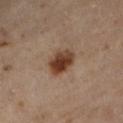image-analysis metrics=an area of roughly 7.5 mm² and a symmetry-axis asymmetry near 0.15; a border-irregularity rating of about 1.5/10; a classifier nevus-likeness of about 100/100 and a lesion-detection confidence of about 100/100 | anatomic site=the left lower leg | patient=female, about 60 years old | lesion size=about 3.5 mm | illumination=cross-polarized | image source=15 mm crop, total-body photography.